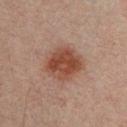| field | value |
|---|---|
| workup | no biopsy performed (imaged during a skin exam) |
| image source | ~15 mm tile from a whole-body skin photo |
| anatomic site | the chest |
| patient | male, about 30 years old |